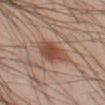Q: Was this lesion biopsied?
A: no biopsy performed (imaged during a skin exam)
Q: What are the patient's age and sex?
A: male, aged approximately 40
Q: Lesion size?
A: ~5 mm (longest diameter)
Q: What is the anatomic site?
A: the leg
Q: What kind of image is this?
A: 15 mm crop, total-body photography
Q: What lighting was used for the tile?
A: cross-polarized illumination